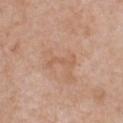On the chest. A female subject, approximately 40 years of age. The recorded lesion diameter is about 3.5 mm. A lesion tile, about 15 mm wide, cut from a 3D total-body photograph. Captured under white-light illumination. The total-body-photography lesion software estimated an area of roughly 3.5 mm² and an outline eccentricity of about 0.95 (0 = round, 1 = elongated). And it measured internal color variation of about 0 on a 0–10 scale and a peripheral color-asymmetry measure near 0.